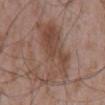Impression:
Captured during whole-body skin photography for melanoma surveillance; the lesion was not biopsied.
Image and clinical context:
On the mid back. Longest diameter approximately 10 mm. A region of skin cropped from a whole-body photographic capture, roughly 15 mm wide. A male subject aged 48 to 52.